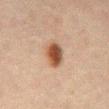Case summary:
- biopsy status · imaged on a skin check; not biopsied
- subject · male, aged around 50
- location · the front of the torso
- image · ~15 mm crop, total-body skin-cancer survey